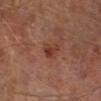| field | value |
|---|---|
| workup | imaged on a skin check; not biopsied |
| illumination | cross-polarized |
| image source | 15 mm crop, total-body photography |
| patient | male, aged 63 to 67 |
| site | the right lower leg |
| lesion diameter | about 2.5 mm |